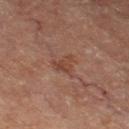No biopsy was performed on this lesion — it was imaged during a full skin examination and was not determined to be concerning.
Imaged with cross-polarized lighting.
The total-body-photography lesion software estimated an eccentricity of roughly 0.45 and a symmetry-axis asymmetry near 0.45. And it measured a normalized lesion–skin contrast near 6. The software also gave border irregularity of about 4.5 on a 0–10 scale and a color-variation rating of about 3/10. And it measured an automated nevus-likeness rating near 0 out of 100 and a detector confidence of about 100 out of 100 that the crop contains a lesion.
On the left thigh.
A roughly 15 mm field-of-view crop from a total-body skin photograph.
A male subject, roughly 70 years of age.